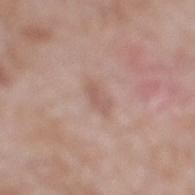<tbp_lesion>
  <biopsy_status>not biopsied; imaged during a skin examination</biopsy_status>
  <lesion_size>
    <long_diameter_mm_approx>3.0</long_diameter_mm_approx>
  </lesion_size>
  <site>right lower leg</site>
  <image>
    <source>total-body photography crop</source>
    <field_of_view_mm>15</field_of_view_mm>
  </image>
  <lighting>white-light</lighting>
  <patient>
    <sex>male</sex>
    <age_approx>60</age_approx>
  </patient>
  <automated_metrics>
    <eccentricity>0.85</eccentricity>
    <shape_asymmetry>0.15</shape_asymmetry>
    <cielab_L>58</cielab_L>
    <cielab_a>18</cielab_a>
    <cielab_b>24</cielab_b>
    <vs_skin_darker_L>7.0</vs_skin_darker_L>
  </automated_metrics>
</tbp_lesion>